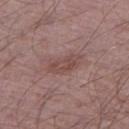The lesion was tiled from a total-body skin photograph and was not biopsied.
A close-up tile cropped from a whole-body skin photograph, about 15 mm across.
A male subject, aged 63–67.
Longest diameter approximately 4.5 mm.
Automated tile analysis of the lesion measured an eccentricity of roughly 0.9. And it measured a classifier nevus-likeness of about 0/100 and lesion-presence confidence of about 100/100.
The tile uses white-light illumination.
The lesion is located on the left thigh.